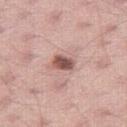Notes:
• follow-up: catalogued during a skin exam; not biopsied
• location: the right thigh
• image source: ~15 mm tile from a whole-body skin photo
• TBP lesion metrics: a footprint of about 4.5 mm², a shape eccentricity near 0.7, and a shape-asymmetry score of about 0.2 (0 = symmetric); a lesion–skin lightness drop of about 16 and a normalized border contrast of about 10.5; internal color variation of about 4.5 on a 0–10 scale and radial color variation of about 1.5
• subject: male, aged around 35
• lesion diameter: ~2.5 mm (longest diameter)